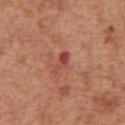Recorded during total-body skin imaging; not selected for excision or biopsy. A male patient about 65 years old. A close-up tile cropped from a whole-body skin photograph, about 15 mm across. On the abdomen. Imaged with white-light lighting. About 3 mm across.